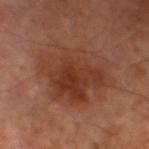{"biopsy_status": "not biopsied; imaged during a skin examination", "image": {"source": "total-body photography crop", "field_of_view_mm": 15}, "automated_metrics": {"cielab_L": 37, "cielab_a": 23, "cielab_b": 29, "vs_skin_darker_L": 8.0, "vs_skin_contrast_norm": 7.0, "nevus_likeness_0_100": 15, "lesion_detection_confidence_0_100": 100}, "patient": {"sex": "male", "age_approx": 70}, "lesion_size": {"long_diameter_mm_approx": 8.0}, "site": "leg"}